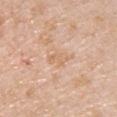Q: Was a biopsy performed?
A: imaged on a skin check; not biopsied
Q: Lesion location?
A: the chest
Q: How large is the lesion?
A: ~2.5 mm (longest diameter)
Q: What are the patient's age and sex?
A: male, aged 48–52
Q: What is the imaging modality?
A: 15 mm crop, total-body photography
Q: What did automated image analysis measure?
A: a mean CIELAB color near L≈67 a*≈19 b*≈34 and a normalized lesion–skin contrast near 4.5
Q: What lighting was used for the tile?
A: white-light